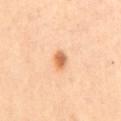Clinical impression:
Part of a total-body skin-imaging series; this lesion was reviewed on a skin check and was not flagged for biopsy.
Image and clinical context:
The lesion is located on the mid back. A male subject aged approximately 55. A 15 mm close-up tile from a total-body photography series done for melanoma screening.